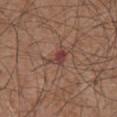subject: male, aged 73 to 77; size: ~2.5 mm (longest diameter); acquisition: ~15 mm tile from a whole-body skin photo; automated metrics: a classifier nevus-likeness of about 0/100; site: the chest.